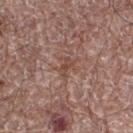Impression:
This lesion was catalogued during total-body skin photography and was not selected for biopsy.
Background:
Automated tile analysis of the lesion measured an average lesion color of about L≈47 a*≈20 b*≈25 (CIELAB) and a normalized lesion–skin contrast near 6. And it measured a border-irregularity index near 4.5/10, internal color variation of about 1 on a 0–10 scale, and radial color variation of about 0. The analysis additionally found a classifier nevus-likeness of about 0/100 and a lesion-detection confidence of about 55/100. A male subject aged 68–72. The lesion's longest dimension is about 3 mm. The tile uses white-light illumination. Located on the right thigh. This image is a 15 mm lesion crop taken from a total-body photograph.